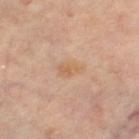Impression:
This lesion was catalogued during total-body skin photography and was not selected for biopsy.
Image and clinical context:
The total-body-photography lesion software estimated border irregularity of about 3.5 on a 0–10 scale, a color-variation rating of about 2/10, and peripheral color asymmetry of about 0.5. Measured at roughly 2.5 mm in maximum diameter. Located on the right leg. This is a cross-polarized tile. This image is a 15 mm lesion crop taken from a total-body photograph. A female subject aged 63–67.